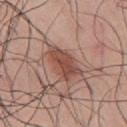Captured during whole-body skin photography for melanoma surveillance; the lesion was not biopsied. The subject is a male in their 40s. The tile uses white-light illumination. The total-body-photography lesion software estimated an average lesion color of about L≈49 a*≈23 b*≈26 (CIELAB), a lesion–skin lightness drop of about 11, and a lesion-to-skin contrast of about 8 (normalized; higher = more distinct). The software also gave a border-irregularity rating of about 3.5/10, a within-lesion color-variation index near 3.5/10, and peripheral color asymmetry of about 1. The lesion is on the mid back. Measured at roughly 5 mm in maximum diameter. A lesion tile, about 15 mm wide, cut from a 3D total-body photograph.